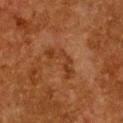Findings:
- workup · imaged on a skin check; not biopsied
- acquisition · ~15 mm crop, total-body skin-cancer survey
- illumination · cross-polarized
- size · ≈4.5 mm
- location · the chest
- patient · female, aged around 50